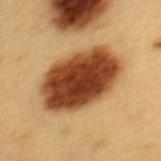| key | value |
|---|---|
| biopsy status | total-body-photography surveillance lesion; no biopsy |
| lesion size | ~8.5 mm (longest diameter) |
| body site | the upper back |
| illumination | cross-polarized illumination |
| image | total-body-photography crop, ~15 mm field of view |
| patient | female, aged approximately 30 |
| image-analysis metrics | a footprint of about 37 mm², an eccentricity of roughly 0.75, and a shape-asymmetry score of about 0.1 (0 = symmetric); a border-irregularity index near 1.5/10 |